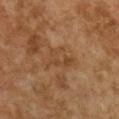Impression: No biopsy was performed on this lesion — it was imaged during a full skin examination and was not determined to be concerning.